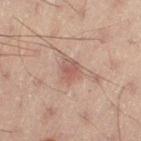Impression: Recorded during total-body skin imaging; not selected for excision or biopsy. Image and clinical context: A male subject, aged 48–52. A 15 mm close-up tile from a total-body photography series done for melanoma screening. The lesion is on the left thigh.